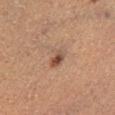This lesion was catalogued during total-body skin photography and was not selected for biopsy. Located on the right lower leg. A roughly 15 mm field-of-view crop from a total-body skin photograph. The lesion-visualizer software estimated two-axis asymmetry of about 0.2. The analysis additionally found internal color variation of about 5.5 on a 0–10 scale and a peripheral color-asymmetry measure near 2. The lesion's longest dimension is about 2.5 mm. The tile uses cross-polarized illumination. A male subject, aged approximately 40.